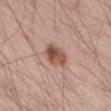Case summary:
– notes · total-body-photography surveillance lesion; no biopsy
– tile lighting · white-light illumination
– imaging modality · total-body-photography crop, ~15 mm field of view
– subject · male, aged 58 to 62
– anatomic site · the left thigh
– diameter · about 3.5 mm